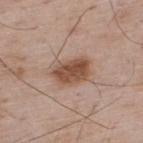An algorithmic analysis of the crop reported a footprint of about 12 mm² and a symmetry-axis asymmetry near 0.2. It also reported border irregularity of about 2.5 on a 0–10 scale, internal color variation of about 4.5 on a 0–10 scale, and a peripheral color-asymmetry measure near 1.5.
Longest diameter approximately 5 mm.
This image is a 15 mm lesion crop taken from a total-body photograph.
The lesion is on the upper back.
The tile uses white-light illumination.
A male patient, aged around 65.